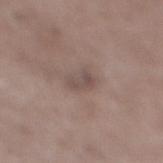This lesion was catalogued during total-body skin photography and was not selected for biopsy. A close-up tile cropped from a whole-body skin photograph, about 15 mm across. The patient is a male aged 48–52. On the right lower leg. About 3 mm across. Imaged with white-light lighting.